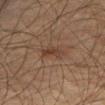Assessment:
Captured during whole-body skin photography for melanoma surveillance; the lesion was not biopsied.
Context:
A 15 mm crop from a total-body photograph taken for skin-cancer surveillance. Automated image analysis of the tile measured a lesion area of about 5.5 mm², a shape eccentricity near 0.9, and two-axis asymmetry of about 0.3. It also reported a classifier nevus-likeness of about 5/100. The tile uses cross-polarized illumination. On the front of the torso. A male patient, aged approximately 70.